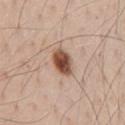patient: male, approximately 40 years of age
lighting: white-light
image source: ~15 mm tile from a whole-body skin photo
TBP lesion metrics: an area of roughly 7 mm², an outline eccentricity of about 0.75 (0 = round, 1 = elongated), and two-axis asymmetry of about 0.2; a nevus-likeness score of about 100/100
site: the chest
lesion size: ~3.5 mm (longest diameter)